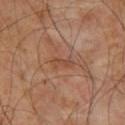Recorded during total-body skin imaging; not selected for excision or biopsy. The lesion is on the chest. A 15 mm crop from a total-body photograph taken for skin-cancer surveillance. Approximately 3 mm at its widest. A male subject aged approximately 60.